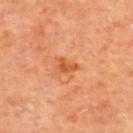Q: Is there a histopathology result?
A: catalogued during a skin exam; not biopsied